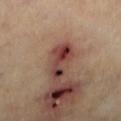Q: Was a biopsy performed?
A: imaged on a skin check; not biopsied
Q: What is the imaging modality?
A: ~15 mm tile from a whole-body skin photo
Q: Who is the patient?
A: about 60 years old
Q: What is the anatomic site?
A: the leg